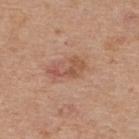<record>
<lighting>white-light</lighting>
<patient>
  <sex>male</sex>
  <age_approx>65</age_approx>
</patient>
<automated_metrics>
  <cielab_L>53</cielab_L>
  <cielab_a>23</cielab_a>
  <cielab_b>30</cielab_b>
  <vs_skin_darker_L>9.0</vs_skin_darker_L>
  <vs_skin_contrast_norm>6.5</vs_skin_contrast_norm>
  <nevus_likeness_0_100>15</nevus_likeness_0_100>
  <lesion_detection_confidence_0_100>100</lesion_detection_confidence_0_100>
</automated_metrics>
<image>
  <source>total-body photography crop</source>
  <field_of_view_mm>15</field_of_view_mm>
</image>
<lesion_size>
  <long_diameter_mm_approx>4.0</long_diameter_mm_approx>
</lesion_size>
<site>upper back</site>
</record>